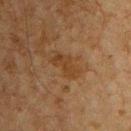The lesion was photographed on a routine skin check and not biopsied; there is no pathology result.
About 4 mm across.
The lesion is on the chest.
A male patient about 65 years old.
An algorithmic analysis of the crop reported an area of roughly 6 mm², an eccentricity of roughly 0.9, and a symmetry-axis asymmetry near 0.35. The analysis additionally found a lesion color around L≈33 a*≈16 b*≈30 in CIELAB, roughly 6 lightness units darker than nearby skin, and a lesion-to-skin contrast of about 6.5 (normalized; higher = more distinct). It also reported a nevus-likeness score of about 0/100.
Imaged with cross-polarized lighting.
Cropped from a whole-body photographic skin survey; the tile spans about 15 mm.